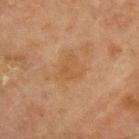The lesion was tiled from a total-body skin photograph and was not biopsied.
An algorithmic analysis of the crop reported about 4 CIELAB-L* units darker than the surrounding skin and a normalized border contrast of about 4.5. And it measured border irregularity of about 4 on a 0–10 scale, a color-variation rating of about 2/10, and radial color variation of about 0.5.
Approximately 3.5 mm at its widest.
On the upper back.
This image is a 15 mm lesion crop taken from a total-body photograph.
The tile uses cross-polarized illumination.
The patient is a male aged 68–72.